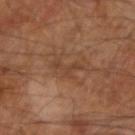Background: The lesion is on the left arm. Automated image analysis of the tile measured an area of roughly 3.5 mm² and an outline eccentricity of about 0.85 (0 = round, 1 = elongated). The software also gave a mean CIELAB color near L≈39 a*≈19 b*≈29, a lesion–skin lightness drop of about 6, and a normalized lesion–skin contrast near 5. The software also gave a border-irregularity rating of about 8.5/10, internal color variation of about 0 on a 0–10 scale, and radial color variation of about 0. The software also gave a detector confidence of about 100 out of 100 that the crop contains a lesion. A region of skin cropped from a whole-body photographic capture, roughly 15 mm wide. The tile uses cross-polarized illumination. Measured at roughly 3.5 mm in maximum diameter. The patient is a male approximately 65 years of age.